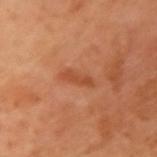{"biopsy_status": "not biopsied; imaged during a skin examination", "image": {"source": "total-body photography crop", "field_of_view_mm": 15}, "patient": {"sex": "female", "age_approx": 55}, "site": "left upper arm", "automated_metrics": {"cielab_L": 51, "cielab_a": 29, "cielab_b": 39, "vs_skin_darker_L": 8.0, "vs_skin_contrast_norm": 6.0}, "lesion_size": {"long_diameter_mm_approx": 3.5}, "lighting": "cross-polarized"}